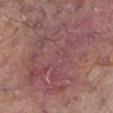biopsy status: no biopsy performed (imaged during a skin exam)
illumination: cross-polarized
location: the left lower leg
diameter: about 11.5 mm
acquisition: ~15 mm tile from a whole-body skin photo
subject: male, approximately 70 years of age
image-analysis metrics: a lesion area of about 55 mm² and a symmetry-axis asymmetry near 0.35; a lesion color around L≈45 a*≈22 b*≈17 in CIELAB, roughly 5 lightness units darker than nearby skin, and a lesion-to-skin contrast of about 5 (normalized; higher = more distinct); a border-irregularity rating of about 6.5/10, a within-lesion color-variation index near 5/10, and radial color variation of about 1.5; a detector confidence of about 70 out of 100 that the crop contains a lesion